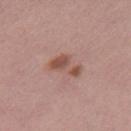workup: no biopsy performed (imaged during a skin exam) | tile lighting: white-light | imaging modality: ~15 mm crop, total-body skin-cancer survey | lesion diameter: about 4 mm | patient: female, roughly 40 years of age | location: the right thigh | automated lesion analysis: an area of roughly 7 mm² and an outline eccentricity of about 0.85 (0 = round, 1 = elongated); a border-irregularity index near 4.5/10 and a peripheral color-asymmetry measure near 1.5; a lesion-detection confidence of about 100/100.